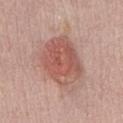- notes — catalogued during a skin exam; not biopsied
- imaging modality — ~15 mm tile from a whole-body skin photo
- site — the abdomen
- patient — male, approximately 60 years of age